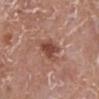Assessment: Captured during whole-body skin photography for melanoma surveillance; the lesion was not biopsied. Image and clinical context: Automated image analysis of the tile measured a mean CIELAB color near L≈46 a*≈24 b*≈27, roughly 11 lightness units darker than nearby skin, and a lesion-to-skin contrast of about 8.5 (normalized; higher = more distinct). It also reported border irregularity of about 4 on a 0–10 scale. And it measured a classifier nevus-likeness of about 80/100 and a lesion-detection confidence of about 100/100. A 15 mm close-up tile from a total-body photography series done for melanoma screening. The lesion's longest dimension is about 3.5 mm. Captured under white-light illumination. From the left lower leg. The patient is a female about 75 years old.